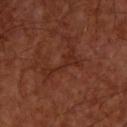The lesion was tiled from a total-body skin photograph and was not biopsied. Cropped from a total-body skin-imaging series; the visible field is about 15 mm. The lesion-visualizer software estimated a border-irregularity rating of about 10/10, internal color variation of about 1.5 on a 0–10 scale, and peripheral color asymmetry of about 0.5. The software also gave a classifier nevus-likeness of about 0/100 and a lesion-detection confidence of about 90/100. On the back. About 4.5 mm across. Imaged with cross-polarized lighting. The patient is a male about 55 years old.